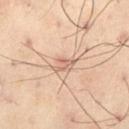Q: Was this lesion biopsied?
A: no biopsy performed (imaged during a skin exam)
Q: What are the patient's age and sex?
A: male, aged 53–57
Q: Lesion location?
A: the left thigh
Q: What kind of image is this?
A: 15 mm crop, total-body photography
Q: How large is the lesion?
A: ≈3 mm